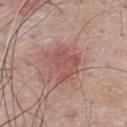Image and clinical context: Cropped from a whole-body photographic skin survey; the tile spans about 15 mm. The lesion is on the upper back. A male patient, aged 43 to 47. The lesion's longest dimension is about 4.5 mm.